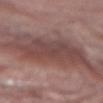This lesion was catalogued during total-body skin photography and was not selected for biopsy. The lesion is on the right forearm. A female patient aged around 70. A 15 mm close-up tile from a total-body photography series done for melanoma screening.